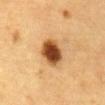The lesion was tiled from a total-body skin photograph and was not biopsied. This is a cross-polarized tile. Located on the abdomen. A roughly 15 mm field-of-view crop from a total-body skin photograph. A male subject aged around 85 or older. About 4.5 mm across. An algorithmic analysis of the crop reported a peripheral color-asymmetry measure near 1. The software also gave a nevus-likeness score of about 100/100 and a lesion-detection confidence of about 100/100.